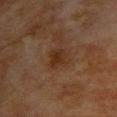follow-up = catalogued during a skin exam; not biopsied | site = the upper back | size = about 3 mm | patient = female, aged 78–82 | automated lesion analysis = a classifier nevus-likeness of about 15/100 | tile lighting = cross-polarized illumination | imaging modality = ~15 mm crop, total-body skin-cancer survey.